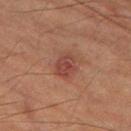| feature | finding |
|---|---|
| notes | imaged on a skin check; not biopsied |
| lesion size | about 3 mm |
| patient | male, aged approximately 85 or older |
| image source | ~15 mm crop, total-body skin-cancer survey |
| location | the left thigh |